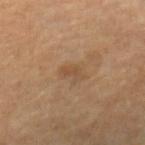No biopsy was performed on this lesion — it was imaged during a full skin examination and was not determined to be concerning. The lesion's longest dimension is about 3 mm. A roughly 15 mm field-of-view crop from a total-body skin photograph. The tile uses cross-polarized illumination. The lesion is on the right lower leg. An algorithmic analysis of the crop reported an area of roughly 3.5 mm². The analysis additionally found a classifier nevus-likeness of about 0/100. A female subject, aged 68–72.